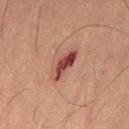The tile uses cross-polarized illumination.
A roughly 15 mm field-of-view crop from a total-body skin photograph.
Located on the left thigh.
Measured at roughly 4.5 mm in maximum diameter.
The patient is a male aged 48–52.